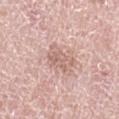Notes:
* workup: catalogued during a skin exam; not biopsied
* automated metrics: an area of roughly 7.5 mm², a shape eccentricity near 0.6, and two-axis asymmetry of about 0.35; a mean CIELAB color near L≈63 a*≈19 b*≈25, about 9 CIELAB-L* units darker than the surrounding skin, and a normalized lesion–skin contrast near 5.5; border irregularity of about 4 on a 0–10 scale, a color-variation rating of about 3/10, and a peripheral color-asymmetry measure near 1
* location: the left thigh
* imaging modality: 15 mm crop, total-body photography
* subject: female, roughly 60 years of age
* diameter: about 3.5 mm